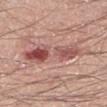Recorded during total-body skin imaging; not selected for excision or biopsy.
A 15 mm crop from a total-body photograph taken for skin-cancer surveillance.
Captured under white-light illumination.
A male patient roughly 40 years of age.
From the leg.